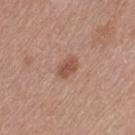Acquisition and patient details: A region of skin cropped from a whole-body photographic capture, roughly 15 mm wide. The lesion-visualizer software estimated a within-lesion color-variation index near 1.5/10. It also reported an automated nevus-likeness rating near 75 out of 100. The tile uses white-light illumination. A female subject, aged approximately 55. The lesion is on the left thigh.